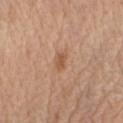The lesion was tiled from a total-body skin photograph and was not biopsied. Located on the right upper arm. A region of skin cropped from a whole-body photographic capture, roughly 15 mm wide. The patient is a male in their 70s. Longest diameter approximately 2.5 mm. Captured under white-light illumination.